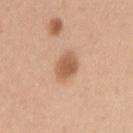Findings:
• follow-up — no biopsy performed (imaged during a skin exam)
• subject — female, aged 23 to 27
• image — 15 mm crop, total-body photography
• location — the arm